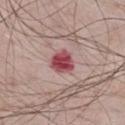Notes:
– follow-up · total-body-photography surveillance lesion; no biopsy
– tile lighting · white-light
– body site · the abdomen
– acquisition · 15 mm crop, total-body photography
– lesion diameter · ~3 mm (longest diameter)
– subject · male, approximately 65 years of age